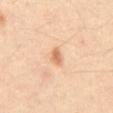{"biopsy_status": "not biopsied; imaged during a skin examination", "site": "abdomen", "automated_metrics": {"eccentricity": 0.65, "shape_asymmetry": 0.25, "nevus_likeness_0_100": 75}, "lighting": "cross-polarized", "lesion_size": {"long_diameter_mm_approx": 2.5}, "image": {"source": "total-body photography crop", "field_of_view_mm": 15}, "patient": {"sex": "male", "age_approx": 60}}